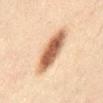No biopsy was performed on this lesion — it was imaged during a full skin examination and was not determined to be concerning. Captured under cross-polarized illumination. Measured at roughly 6 mm in maximum diameter. The lesion is on the abdomen. A male subject aged around 50. This image is a 15 mm lesion crop taken from a total-body photograph.